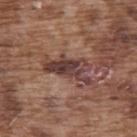Clinical impression: The lesion was photographed on a routine skin check and not biopsied; there is no pathology result. Background: A region of skin cropped from a whole-body photographic capture, roughly 15 mm wide. The lesion is on the upper back. A male subject in their mid- to late 70s.